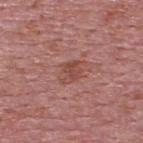Captured during whole-body skin photography for melanoma surveillance; the lesion was not biopsied. A 15 mm close-up extracted from a 3D total-body photography capture. An algorithmic analysis of the crop reported a mean CIELAB color near L≈48 a*≈25 b*≈26, roughly 7 lightness units darker than nearby skin, and a normalized lesion–skin contrast near 6. The software also gave a classifier nevus-likeness of about 0/100 and a lesion-detection confidence of about 100/100. The lesion is on the upper back. A male subject, roughly 75 years of age. Captured under white-light illumination.